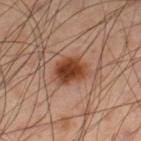Clinical impression:
No biopsy was performed on this lesion — it was imaged during a full skin examination and was not determined to be concerning.
Acquisition and patient details:
The recorded lesion diameter is about 3.5 mm. This image is a 15 mm lesion crop taken from a total-body photograph. The patient is a male approximately 55 years of age. An algorithmic analysis of the crop reported a lesion color around L≈42 a*≈25 b*≈33 in CIELAB, a lesion–skin lightness drop of about 14, and a normalized lesion–skin contrast near 11.5. On the right lower leg. Imaged with cross-polarized lighting.